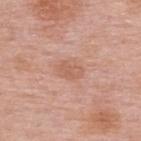Q: Was a biopsy performed?
A: imaged on a skin check; not biopsied
Q: Illumination type?
A: white-light illumination
Q: Who is the patient?
A: female, in their 50s
Q: What is the imaging modality?
A: 15 mm crop, total-body photography
Q: How large is the lesion?
A: about 3 mm
Q: Where on the body is the lesion?
A: the upper back